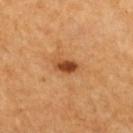workup — imaged on a skin check; not biopsied | patient — female, aged 63–67 | acquisition — total-body-photography crop, ~15 mm field of view | lighting — cross-polarized | site — the upper back.